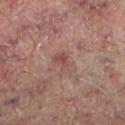Assessment: Imaged during a routine full-body skin examination; the lesion was not biopsied and no histopathology is available. Background: The total-body-photography lesion software estimated a border-irregularity index near 3.5/10, a color-variation rating of about 3.5/10, and a peripheral color-asymmetry measure near 1. Cropped from a total-body skin-imaging series; the visible field is about 15 mm. The subject is a male about 70 years old. Captured under cross-polarized illumination. From the left lower leg. Longest diameter approximately 3.5 mm.